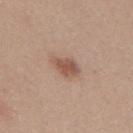notes = catalogued during a skin exam; not biopsied
lesion size = about 3.5 mm
acquisition = ~15 mm tile from a whole-body skin photo
automated lesion analysis = an eccentricity of roughly 0.8 and a symmetry-axis asymmetry near 0.25; lesion-presence confidence of about 100/100
patient = male, aged 38–42
anatomic site = the mid back
illumination = white-light